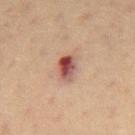workup — total-body-photography surveillance lesion; no biopsy | automated lesion analysis — a lesion area of about 5.5 mm², a shape eccentricity near 0.8, and two-axis asymmetry of about 0.2; an automated nevus-likeness rating near 0 out of 100 and a lesion-detection confidence of about 100/100 | size — ~3 mm (longest diameter) | subject — male, aged 68 to 72 | acquisition — ~15 mm crop, total-body skin-cancer survey | location — the front of the torso | tile lighting — cross-polarized.